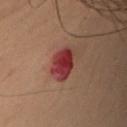| field | value |
|---|---|
| image-analysis metrics | an area of roughly 8 mm², a shape eccentricity near 0.5, and a shape-asymmetry score of about 0.2 (0 = symmetric) |
| subject | male, about 55 years old |
| size | ≈3 mm |
| site | the left upper arm |
| tile lighting | cross-polarized illumination |
| image source | 15 mm crop, total-body photography |